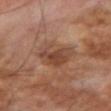Q: Was a biopsy performed?
A: total-body-photography surveillance lesion; no biopsy
Q: What are the patient's age and sex?
A: male, in their 70s
Q: Where on the body is the lesion?
A: the right upper arm
Q: Automated lesion metrics?
A: an area of roughly 12 mm² and an eccentricity of roughly 0.55; a border-irregularity rating of about 4/10 and a peripheral color-asymmetry measure near 1.5; an automated nevus-likeness rating near 10 out of 100 and lesion-presence confidence of about 100/100
Q: Illumination type?
A: cross-polarized illumination
Q: How large is the lesion?
A: about 5 mm
Q: What is the imaging modality?
A: ~15 mm crop, total-body skin-cancer survey